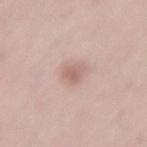Imaged during a routine full-body skin examination; the lesion was not biopsied and no histopathology is available. A 15 mm crop from a total-body photograph taken for skin-cancer surveillance. A male subject, about 60 years old. Approximately 3 mm at its widest. The lesion is on the back. The lesion-visualizer software estimated a lesion area of about 4.5 mm², an outline eccentricity of about 0.8 (0 = round, 1 = elongated), and two-axis asymmetry of about 0.3. It also reported a normalized lesion–skin contrast near 6.